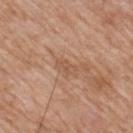Q: Was a biopsy performed?
A: catalogued during a skin exam; not biopsied
Q: Lesion location?
A: the mid back
Q: What is the imaging modality?
A: 15 mm crop, total-body photography
Q: What are the patient's age and sex?
A: male, aged around 60